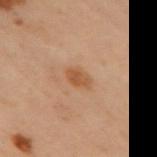The lesion was tiled from a total-body skin photograph and was not biopsied.
A roughly 15 mm field-of-view crop from a total-body skin photograph.
From the upper back.
A female subject, about 60 years old.
The recorded lesion diameter is about 2.5 mm.
An algorithmic analysis of the crop reported about 8 CIELAB-L* units darker than the surrounding skin and a lesion-to-skin contrast of about 7 (normalized; higher = more distinct). The software also gave an automated nevus-likeness rating near 70 out of 100.
This is a cross-polarized tile.